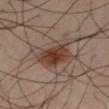Captured during whole-body skin photography for melanoma surveillance; the lesion was not biopsied. The patient is a male aged 38–42. Located on the leg. The tile uses cross-polarized illumination. Cropped from a whole-body photographic skin survey; the tile spans about 15 mm.